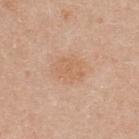Recorded during total-body skin imaging; not selected for excision or biopsy.
A female subject in their 40s.
On the upper back.
A lesion tile, about 15 mm wide, cut from a 3D total-body photograph.